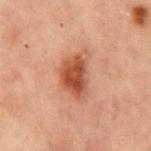No biopsy was performed on this lesion — it was imaged during a full skin examination and was not determined to be concerning.
Measured at roughly 5 mm in maximum diameter.
A roughly 15 mm field-of-view crop from a total-body skin photograph.
On the front of the torso.
A male subject, aged 53–57.